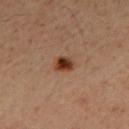Clinical impression: The lesion was tiled from a total-body skin photograph and was not biopsied. Background: Located on the mid back. The subject is a male in their 50s. An algorithmic analysis of the crop reported border irregularity of about 1.5 on a 0–10 scale, internal color variation of about 5 on a 0–10 scale, and a peripheral color-asymmetry measure near 1.5. This image is a 15 mm lesion crop taken from a total-body photograph. Imaged with cross-polarized lighting.